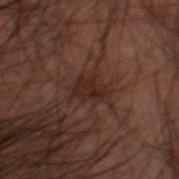Part of a total-body skin-imaging series; this lesion was reviewed on a skin check and was not flagged for biopsy.
The lesion is located on the head or neck.
Automated tile analysis of the lesion measured a border-irregularity index near 4.5/10, a within-lesion color-variation index near 2/10, and peripheral color asymmetry of about 0.5. The software also gave a nevus-likeness score of about 0/100.
A close-up tile cropped from a whole-body skin photograph, about 15 mm across.
The patient is a male in their 30s.
Imaged with cross-polarized lighting.
The lesion's longest dimension is about 4 mm.